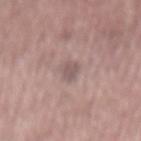follow-up: total-body-photography surveillance lesion; no biopsy | image source: total-body-photography crop, ~15 mm field of view | diameter: ~3 mm (longest diameter) | subject: male, aged around 60 | body site: the mid back | TBP lesion metrics: an area of roughly 3.5 mm², an outline eccentricity of about 0.85 (0 = round, 1 = elongated), and a symmetry-axis asymmetry near 0.35; an average lesion color of about L≈54 a*≈16 b*≈18 (CIELAB) and about 9 CIELAB-L* units darker than the surrounding skin; border irregularity of about 3 on a 0–10 scale, a color-variation rating of about 1.5/10, and a peripheral color-asymmetry measure near 0.5 | tile lighting: white-light illumination.